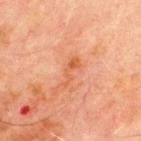Case summary:
– biopsy status — imaged on a skin check; not biopsied
– lighting — cross-polarized illumination
– subject — male, about 70 years old
– acquisition — ~15 mm tile from a whole-body skin photo
– lesion diameter — ~3.5 mm (longest diameter)
– body site — the chest
– automated lesion analysis — a footprint of about 4 mm², an outline eccentricity of about 0.95 (0 = round, 1 = elongated), and a shape-asymmetry score of about 0.45 (0 = symmetric); a lesion color around L≈49 a*≈26 b*≈35 in CIELAB and roughly 7 lightness units darker than nearby skin; a nevus-likeness score of about 0/100 and a detector confidence of about 100 out of 100 that the crop contains a lesion